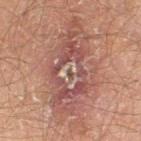Clinical impression: Captured during whole-body skin photography for melanoma surveillance; the lesion was not biopsied. Image and clinical context: Imaged with cross-polarized lighting. Approximately 11 mm at its widest. On the right thigh. A male patient about 60 years old. A 15 mm close-up extracted from a 3D total-body photography capture.